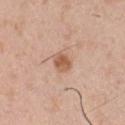{"biopsy_status": "not biopsied; imaged during a skin examination", "patient": {"sex": "male", "age_approx": 30}, "image": {"source": "total-body photography crop", "field_of_view_mm": 15}, "automated_metrics": {"area_mm2_approx": 4.5, "eccentricity": 0.6, "cielab_L": 58, "cielab_a": 21, "cielab_b": 32, "vs_skin_contrast_norm": 8.0, "peripheral_color_asymmetry": 1.0}, "lighting": "white-light", "site": "chest"}